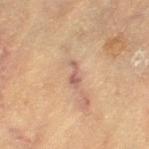follow-up: total-body-photography surveillance lesion; no biopsy
location: the left thigh
illumination: cross-polarized
imaging modality: total-body-photography crop, ~15 mm field of view
patient: female, aged around 80
lesion size: ≈3 mm